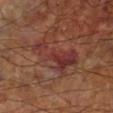Clinical impression:
The lesion was tiled from a total-body skin photograph and was not biopsied.